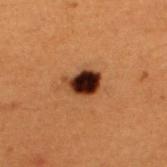Assessment:
Recorded during total-body skin imaging; not selected for excision or biopsy.
Image and clinical context:
The lesion-visualizer software estimated border irregularity of about 2.5 on a 0–10 scale, a within-lesion color-variation index near 10/10, and peripheral color asymmetry of about 3. And it measured an automated nevus-likeness rating near 100 out of 100 and a detector confidence of about 100 out of 100 that the crop contains a lesion. A male subject aged 48 to 52. A region of skin cropped from a whole-body photographic capture, roughly 15 mm wide. Captured under cross-polarized illumination. The recorded lesion diameter is about 3.5 mm. From the upper back.